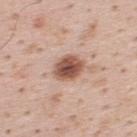No biopsy was performed on this lesion — it was imaged during a full skin examination and was not determined to be concerning. Captured under white-light illumination. On the upper back. The patient is a male in their mid- to late 30s. Automated tile analysis of the lesion measured a border-irregularity index near 1.5/10, a color-variation rating of about 5/10, and a peripheral color-asymmetry measure near 1.5. A close-up tile cropped from a whole-body skin photograph, about 15 mm across.